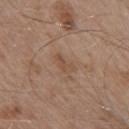Automated image analysis of the tile measured an area of roughly 3 mm², an outline eccentricity of about 0.9 (0 = round, 1 = elongated), and a shape-asymmetry score of about 0.45 (0 = symmetric). The software also gave roughly 6 lightness units darker than nearby skin. And it measured border irregularity of about 5 on a 0–10 scale and peripheral color asymmetry of about 0. The patient is a male in their mid-50s. A lesion tile, about 15 mm wide, cut from a 3D total-body photograph. About 3 mm across. The lesion is located on the right upper arm. This is a white-light tile.